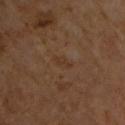This lesion was catalogued during total-body skin photography and was not selected for biopsy.
A male patient, aged around 65.
On the front of the torso.
A close-up tile cropped from a whole-body skin photograph, about 15 mm across.